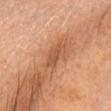<case>
<image>
  <source>total-body photography crop</source>
  <field_of_view_mm>15</field_of_view_mm>
</image>
<patient>
  <sex>female</sex>
  <age_approx>65</age_approx>
</patient>
<site>head or neck</site>
<lighting>cross-polarized</lighting>
<lesion_size>
  <long_diameter_mm_approx>3.5</long_diameter_mm_approx>
</lesion_size>
</case>